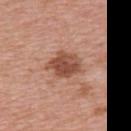The lesion was photographed on a routine skin check and not biopsied; there is no pathology result.
The tile uses white-light illumination.
A 15 mm close-up extracted from a 3D total-body photography capture.
On the upper back.
The lesion-visualizer software estimated a shape-asymmetry score of about 0.25 (0 = symmetric). The analysis additionally found an average lesion color of about L≈50 a*≈24 b*≈30 (CIELAB) and a normalized lesion–skin contrast near 9. The analysis additionally found a border-irregularity index near 2.5/10, a color-variation rating of about 4/10, and radial color variation of about 1.5. It also reported an automated nevus-likeness rating near 85 out of 100 and a lesion-detection confidence of about 100/100.
Approximately 4.5 mm at its widest.
A female patient aged approximately 40.